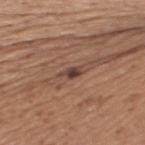Imaged during a routine full-body skin examination; the lesion was not biopsied and no histopathology is available.
Cropped from a total-body skin-imaging series; the visible field is about 15 mm.
Located on the upper back.
The patient is a female aged around 50.